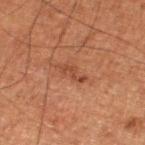A region of skin cropped from a whole-body photographic capture, roughly 15 mm wide.
From the left lower leg.
The subject is a male roughly 75 years of age.